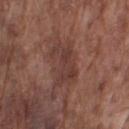Assessment:
This lesion was catalogued during total-body skin photography and was not selected for biopsy.
Context:
A male subject, approximately 75 years of age. Measured at roughly 5 mm in maximum diameter. The tile uses white-light illumination. The lesion is on the right upper arm. Automated image analysis of the tile measured a lesion color around L≈38 a*≈20 b*≈22 in CIELAB and a lesion–skin lightness drop of about 7. A 15 mm close-up extracted from a 3D total-body photography capture.